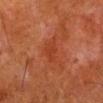Imaged during a routine full-body skin examination; the lesion was not biopsied and no histopathology is available.